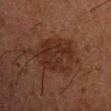Captured during whole-body skin photography for melanoma surveillance; the lesion was not biopsied. Automated image analysis of the tile measured an area of roughly 19 mm² and a symmetry-axis asymmetry near 0.15. And it measured a mean CIELAB color near L≈22 a*≈17 b*≈21, roughly 6 lightness units darker than nearby skin, and a lesion-to-skin contrast of about 7 (normalized; higher = more distinct). From the right upper arm. The patient is a male in their 50s. Captured under cross-polarized illumination. A region of skin cropped from a whole-body photographic capture, roughly 15 mm wide. Longest diameter approximately 6 mm.